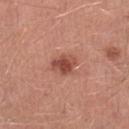Imaged during a routine full-body skin examination; the lesion was not biopsied and no histopathology is available. Located on the left lower leg. A 15 mm close-up tile from a total-body photography series done for melanoma screening. A male subject approximately 45 years of age. This is a white-light tile. The lesion's longest dimension is about 3.5 mm.